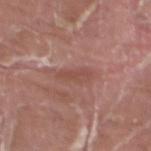automated lesion analysis = a footprint of about 4.5 mm² and a shape eccentricity near 0.9; a border-irregularity rating of about 3/10 | anatomic site = the leg | patient = male, aged 38 to 42 | lighting = white-light | lesion size = ≈3.5 mm | image = ~15 mm crop, total-body skin-cancer survey.